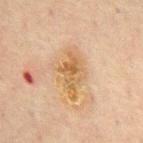Imaged during a routine full-body skin examination; the lesion was not biopsied and no histopathology is available. The subject is a male aged 68 to 72. The lesion is on the back. Captured under cross-polarized illumination. A region of skin cropped from a whole-body photographic capture, roughly 15 mm wide. Automated image analysis of the tile measured an area of roughly 13 mm², an outline eccentricity of about 0.8 (0 = round, 1 = elongated), and a shape-asymmetry score of about 0.35 (0 = symmetric). The analysis additionally found internal color variation of about 5 on a 0–10 scale and radial color variation of about 1.5.